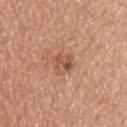Assessment:
This lesion was catalogued during total-body skin photography and was not selected for biopsy.
Background:
This image is a 15 mm lesion crop taken from a total-body photograph. On the upper back. This is a white-light tile. About 2.5 mm across. Automated image analysis of the tile measured a lesion area of about 5.5 mm² and a shape eccentricity near 0.5. The analysis additionally found about 9 CIELAB-L* units darker than the surrounding skin and a lesion-to-skin contrast of about 6 (normalized; higher = more distinct). The patient is a male aged around 50.